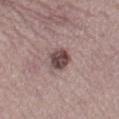This lesion was catalogued during total-body skin photography and was not selected for biopsy. A 15 mm crop from a total-body photograph taken for skin-cancer surveillance. The lesion is located on the left lower leg. About 3 mm across. The tile uses white-light illumination. A female patient aged 48 to 52.